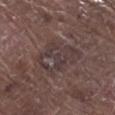Q: Was a biopsy performed?
A: imaged on a skin check; not biopsied
Q: Lesion location?
A: the leg
Q: What lighting was used for the tile?
A: white-light
Q: What is the lesion's diameter?
A: ≈5.5 mm
Q: What kind of image is this?
A: 15 mm crop, total-body photography
Q: Patient demographics?
A: male, aged approximately 80